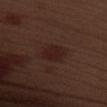Assessment: No biopsy was performed on this lesion — it was imaged during a full skin examination and was not determined to be concerning. Image and clinical context: Approximately 3.5 mm at its widest. The lesion-visualizer software estimated an eccentricity of roughly 0.75 and two-axis asymmetry of about 0.15. It also reported an average lesion color of about L≈21 a*≈19 b*≈20 (CIELAB), a lesion–skin lightness drop of about 6, and a normalized lesion–skin contrast near 7. And it measured a border-irregularity rating of about 1.5/10. A region of skin cropped from a whole-body photographic capture, roughly 15 mm wide. The patient is a male aged approximately 70. The lesion is located on the left upper arm. Captured under white-light illumination.